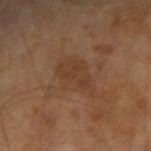<tbp_lesion>
  <biopsy_status>not biopsied; imaged during a skin examination</biopsy_status>
  <image>
    <source>total-body photography crop</source>
    <field_of_view_mm>15</field_of_view_mm>
  </image>
  <site>left arm</site>
  <patient>
    <sex>male</sex>
    <age_approx>65</age_approx>
  </patient>
</tbp_lesion>